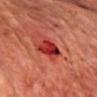The lesion was photographed on a routine skin check and not biopsied; there is no pathology result.
A 15 mm crop from a total-body photograph taken for skin-cancer surveillance.
From the chest.
A male patient, in their 60s.
Automated image analysis of the tile measured an area of roughly 7 mm², an outline eccentricity of about 0.6 (0 = round, 1 = elongated), and two-axis asymmetry of about 0.25. The software also gave roughly 13 lightness units darker than nearby skin and a normalized border contrast of about 10.5. The analysis additionally found a border-irregularity index near 2.5/10 and peripheral color asymmetry of about 2. The software also gave a nevus-likeness score of about 0/100 and lesion-presence confidence of about 100/100.
The tile uses cross-polarized illumination.
Measured at roughly 3.5 mm in maximum diameter.